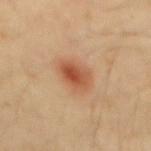workup=no biopsy performed (imaged during a skin exam)
subject=male, aged 28–32
anatomic site=the mid back
image source=~15 mm crop, total-body skin-cancer survey
lesion size=≈3.5 mm
lighting=cross-polarized
automated lesion analysis=an average lesion color of about L≈51 a*≈25 b*≈35 (CIELAB) and a normalized lesion–skin contrast near 8; an automated nevus-likeness rating near 100 out of 100 and lesion-presence confidence of about 100/100